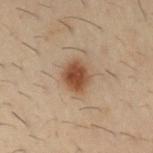The lesion was photographed on a routine skin check and not biopsied; there is no pathology result.
Located on the arm.
Approximately 3 mm at its widest.
A male subject, aged 28 to 32.
A 15 mm close-up tile from a total-body photography series done for melanoma screening.
Imaged with cross-polarized lighting.